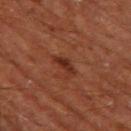The lesion was photographed on a routine skin check and not biopsied; there is no pathology result. A close-up tile cropped from a whole-body skin photograph, about 15 mm across. A male patient, aged around 65. Automated tile analysis of the lesion measured a mean CIELAB color near L≈30 a*≈25 b*≈29 and about 8 CIELAB-L* units darker than the surrounding skin. And it measured border irregularity of about 4 on a 0–10 scale, internal color variation of about 2.5 on a 0–10 scale, and a peripheral color-asymmetry measure near 0.5. The analysis additionally found lesion-presence confidence of about 100/100. This is a cross-polarized tile. The lesion is on the left thigh.